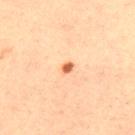<lesion>
<biopsy_status>not biopsied; imaged during a skin examination</biopsy_status>
<lesion_size>
  <long_diameter_mm_approx>1.5</long_diameter_mm_approx>
</lesion_size>
<patient>
  <sex>male</sex>
  <age_approx>30</age_approx>
</patient>
<lighting>cross-polarized</lighting>
<site>upper back</site>
<image>
  <source>total-body photography crop</source>
  <field_of_view_mm>15</field_of_view_mm>
</image>
<automated_metrics>
  <eccentricity>0.65</eccentricity>
  <shape_asymmetry>0.3</shape_asymmetry>
  <cielab_L>54</cielab_L>
  <cielab_a>26</cielab_a>
  <cielab_b>36</cielab_b>
  <vs_skin_darker_L>14.0</vs_skin_darker_L>
  <vs_skin_contrast_norm>10.0</vs_skin_contrast_norm>
</automated_metrics>
</lesion>